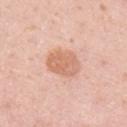Findings:
- notes: no biopsy performed (imaged during a skin exam)
- image source: 15 mm crop, total-body photography
- tile lighting: white-light
- location: the right upper arm
- automated lesion analysis: a mean CIELAB color near L≈66 a*≈24 b*≈32, a lesion–skin lightness drop of about 10, and a normalized lesion–skin contrast near 7; border irregularity of about 1.5 on a 0–10 scale, internal color variation of about 3.5 on a 0–10 scale, and peripheral color asymmetry of about 1
- patient: female, approximately 30 years of age